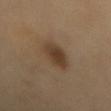Clinical impression: Recorded during total-body skin imaging; not selected for excision or biopsy. Acquisition and patient details: On the mid back. Automated tile analysis of the lesion measured an average lesion color of about L≈40 a*≈15 b*≈28 (CIELAB) and a normalized lesion–skin contrast near 8.5. And it measured an automated nevus-likeness rating near 95 out of 100. A female patient aged 68–72. A 15 mm crop from a total-body photograph taken for skin-cancer surveillance. Captured under cross-polarized illumination. Longest diameter approximately 5 mm.